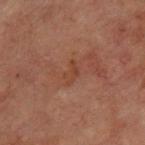Impression: Recorded during total-body skin imaging; not selected for excision or biopsy. Clinical summary: A male patient roughly 70 years of age. This is a cross-polarized tile. The lesion-visualizer software estimated a footprint of about 2.5 mm², an outline eccentricity of about 0.85 (0 = round, 1 = elongated), and a symmetry-axis asymmetry near 0.45. And it measured about 4 CIELAB-L* units darker than the surrounding skin. A 15 mm crop from a total-body photograph taken for skin-cancer surveillance. From the chest.